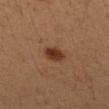Impression:
Recorded during total-body skin imaging; not selected for excision or biopsy.